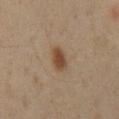This lesion was catalogued during total-body skin photography and was not selected for biopsy. This is a cross-polarized tile. Automated tile analysis of the lesion measured a lesion color around L≈41 a*≈16 b*≈28 in CIELAB, about 10 CIELAB-L* units darker than the surrounding skin, and a lesion-to-skin contrast of about 9 (normalized; higher = more distinct). The software also gave a within-lesion color-variation index near 2.5/10 and peripheral color asymmetry of about 1. It also reported lesion-presence confidence of about 100/100. Located on the abdomen. This image is a 15 mm lesion crop taken from a total-body photograph. A male subject, aged 58–62.